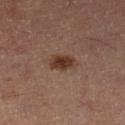The lesion was tiled from a total-body skin photograph and was not biopsied.